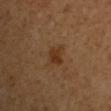No biopsy was performed on this lesion — it was imaged during a full skin examination and was not determined to be concerning.
A close-up tile cropped from a whole-body skin photograph, about 15 mm across.
On the right upper arm.
Automated tile analysis of the lesion measured an automated nevus-likeness rating near 90 out of 100 and a detector confidence of about 100 out of 100 that the crop contains a lesion.
The patient is a male about 50 years old.
The tile uses cross-polarized illumination.